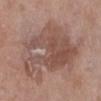| field | value |
|---|---|
| biopsy status | catalogued during a skin exam; not biopsied |
| lesion diameter | ≈9.5 mm |
| subject | female, aged 68–72 |
| imaging modality | ~15 mm crop, total-body skin-cancer survey |
| site | the left lower leg |
| illumination | white-light illumination |
| TBP lesion metrics | a lesion area of about 35 mm², an eccentricity of roughly 0.7, and a symmetry-axis asymmetry near 0.45; a border-irregularity index near 8.5/10 and peripheral color asymmetry of about 1.5 |